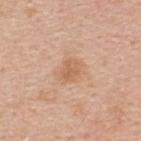follow-up = imaged on a skin check; not biopsied.